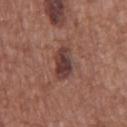site: the chest | patient: male, approximately 75 years of age | image-analysis metrics: a lesion area of about 8 mm² and an eccentricity of roughly 0.75; a mean CIELAB color near L≈39 a*≈21 b*≈21, a lesion–skin lightness drop of about 11, and a normalized lesion–skin contrast near 9.5; a border-irregularity rating of about 2/10, a within-lesion color-variation index near 5/10, and radial color variation of about 1.5; a classifier nevus-likeness of about 60/100 and a lesion-detection confidence of about 100/100 | lesion size: ~3.5 mm (longest diameter) | image source: total-body-photography crop, ~15 mm field of view | tile lighting: white-light illumination.